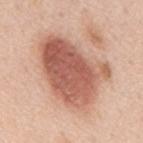The lesion was tiled from a total-body skin photograph and was not biopsied. A male patient roughly 50 years of age. The lesion-visualizer software estimated a border-irregularity rating of about 3.5/10, internal color variation of about 6.5 on a 0–10 scale, and a peripheral color-asymmetry measure near 2.5. Located on the mid back. A region of skin cropped from a whole-body photographic capture, roughly 15 mm wide. Approximately 8.5 mm at its widest.